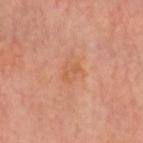Imaged during a routine full-body skin examination; the lesion was not biopsied and no histopathology is available. A 15 mm close-up extracted from a 3D total-body photography capture. The total-body-photography lesion software estimated a color-variation rating of about 2.5/10 and a peripheral color-asymmetry measure near 1. It also reported an automated nevus-likeness rating near 0 out of 100 and a detector confidence of about 100 out of 100 that the crop contains a lesion. A male patient, aged approximately 65. Captured under cross-polarized illumination. The lesion is on the head or neck.